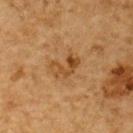Q: Was a biopsy performed?
A: no biopsy performed (imaged during a skin exam)
Q: Patient demographics?
A: male, aged 83 or over
Q: What did automated image analysis measure?
A: a shape eccentricity near 0.8 and a shape-asymmetry score of about 0.5 (0 = symmetric); a within-lesion color-variation index near 6.5/10 and peripheral color asymmetry of about 2.5; an automated nevus-likeness rating near 25 out of 100 and a lesion-detection confidence of about 100/100
Q: What is the lesion's diameter?
A: ~3.5 mm (longest diameter)
Q: Illumination type?
A: cross-polarized
Q: Lesion location?
A: the upper back
Q: What is the imaging modality?
A: ~15 mm tile from a whole-body skin photo